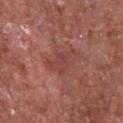notes — total-body-photography surveillance lesion; no biopsy
patient — male, in their mid- to late 60s
tile lighting — white-light illumination
body site — the chest
TBP lesion metrics — a footprint of about 8 mm² and a shape-asymmetry score of about 0.3 (0 = symmetric); a mean CIELAB color near L≈44 a*≈26 b*≈25 and a lesion–skin lightness drop of about 6; a border-irregularity rating of about 4/10, a color-variation rating of about 2.5/10, and peripheral color asymmetry of about 1
lesion diameter — about 4 mm
imaging modality — ~15 mm tile from a whole-body skin photo